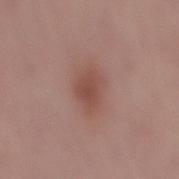Impression: The lesion was tiled from a total-body skin photograph and was not biopsied. Background: Located on the mid back. A male subject aged 53 to 57. A roughly 15 mm field-of-view crop from a total-body skin photograph.